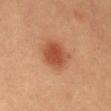The tile uses cross-polarized illumination.
The subject is a female approximately 60 years of age.
The total-body-photography lesion software estimated a lesion color around L≈44 a*≈25 b*≈32 in CIELAB, roughly 10 lightness units darker than nearby skin, and a normalized border contrast of about 8. It also reported a nevus-likeness score of about 100/100.
This image is a 15 mm lesion crop taken from a total-body photograph.
Located on the mid back.